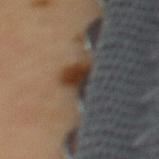Clinical impression:
Imaged during a routine full-body skin examination; the lesion was not biopsied and no histopathology is available.
Acquisition and patient details:
The lesion is on the mid back. The tile uses cross-polarized illumination. A region of skin cropped from a whole-body photographic capture, roughly 15 mm wide. A female subject, approximately 50 years of age.